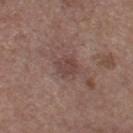Findings:
- workup · imaged on a skin check; not biopsied
- imaging modality · ~15 mm crop, total-body skin-cancer survey
- diameter · ~2.5 mm (longest diameter)
- automated lesion analysis · a lesion area of about 5.5 mm², an eccentricity of roughly 0.6, and two-axis asymmetry of about 0.25; a mean CIELAB color near L≈43 a*≈18 b*≈21, about 7 CIELAB-L* units darker than the surrounding skin, and a lesion-to-skin contrast of about 6 (normalized; higher = more distinct)
- body site · the left thigh
- lighting · white-light illumination
- subject · female, aged 63 to 67